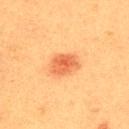  biopsy_status: not biopsied; imaged during a skin examination
  image:
    source: total-body photography crop
    field_of_view_mm: 15
  patient:
    sex: male
    age_approx: 40
  lesion_size:
    long_diameter_mm_approx: 3.5
  site: upper back
  automated_metrics:
    cielab_L: 54
    cielab_a: 28
    cielab_b: 38
    vs_skin_darker_L: 11.0
    vs_skin_contrast_norm: 7.0
    color_variation_0_10: 4.0